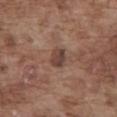The lesion was tiled from a total-body skin photograph and was not biopsied. Captured under white-light illumination. Approximately 2.5 mm at its widest. A 15 mm crop from a total-body photograph taken for skin-cancer surveillance. A male subject in their mid- to late 70s. Located on the abdomen.